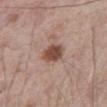  biopsy_status: not biopsied; imaged during a skin examination
  lesion_size:
    long_diameter_mm_approx: 3.0
  site: front of the torso
  lighting: white-light
  image:
    source: total-body photography crop
    field_of_view_mm: 15
  patient:
    sex: male
    age_approx: 80